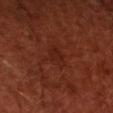The lesion was photographed on a routine skin check and not biopsied; there is no pathology result.
Captured under cross-polarized illumination.
The subject is a male aged around 50.
From the head or neck.
Approximately 3 mm at its widest.
The lesion-visualizer software estimated border irregularity of about 3.5 on a 0–10 scale and peripheral color asymmetry of about 0.5. The software also gave an automated nevus-likeness rating near 0 out of 100 and a lesion-detection confidence of about 100/100.
This image is a 15 mm lesion crop taken from a total-body photograph.